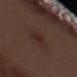Q: Was a biopsy performed?
A: catalogued during a skin exam; not biopsied
Q: What did automated image analysis measure?
A: a lesion area of about 5 mm², a shape eccentricity near 0.75, and a shape-asymmetry score of about 0.2 (0 = symmetric)
Q: What is the anatomic site?
A: the left forearm
Q: What are the patient's age and sex?
A: male, aged 68–72
Q: What is the lesion's diameter?
A: about 3.5 mm
Q: What lighting was used for the tile?
A: white-light illumination
Q: What is the imaging modality?
A: 15 mm crop, total-body photography